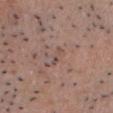{"biopsy_status": "not biopsied; imaged during a skin examination", "site": "head or neck", "image": {"source": "total-body photography crop", "field_of_view_mm": 15}, "lesion_size": {"long_diameter_mm_approx": 2.5}, "lighting": "white-light", "patient": {"sex": "male", "age_approx": 50}, "automated_metrics": {"vs_skin_contrast_norm": 5.0, "border_irregularity_0_10": 4.5, "color_variation_0_10": 0.0, "peripheral_color_asymmetry": 0.0}}